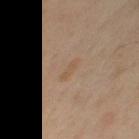Q: What is the imaging modality?
A: total-body-photography crop, ~15 mm field of view
Q: Where on the body is the lesion?
A: the front of the torso
Q: What are the patient's age and sex?
A: male, aged 63 to 67